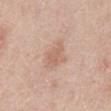The lesion was tiled from a total-body skin photograph and was not biopsied. The lesion's longest dimension is about 2.5 mm. Captured under white-light illumination. A lesion tile, about 15 mm wide, cut from a 3D total-body photograph. The lesion is located on the mid back. A male subject in their 40s. Automated image analysis of the tile measured an area of roughly 4.5 mm² and two-axis asymmetry of about 0.4. And it measured a border-irregularity rating of about 4/10, internal color variation of about 1 on a 0–10 scale, and radial color variation of about 0.5.